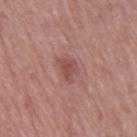Part of a total-body skin-imaging series; this lesion was reviewed on a skin check and was not flagged for biopsy. From the leg. A lesion tile, about 15 mm wide, cut from a 3D total-body photograph. The lesion-visualizer software estimated roughly 8 lightness units darker than nearby skin and a normalized lesion–skin contrast near 6. A female subject approximately 65 years of age. About 3 mm across. The tile uses white-light illumination.